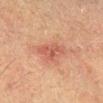Q: Is there a histopathology result?
A: imaged on a skin check; not biopsied
Q: What kind of image is this?
A: ~15 mm crop, total-body skin-cancer survey
Q: Who is the patient?
A: male, aged around 60
Q: Where on the body is the lesion?
A: the right lower leg
Q: Lesion size?
A: about 3.5 mm